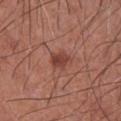Imaged with white-light lighting.
The subject is a male in their mid- to late 60s.
This image is a 15 mm lesion crop taken from a total-body photograph.
Located on the chest.
The lesion's longest dimension is about 3 mm.